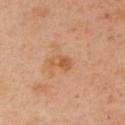Captured during whole-body skin photography for melanoma surveillance; the lesion was not biopsied.
The total-body-photography lesion software estimated an outline eccentricity of about 0.75 (0 = round, 1 = elongated). The analysis additionally found an average lesion color of about L≈58 a*≈24 b*≈38 (CIELAB), roughly 8 lightness units darker than nearby skin, and a lesion-to-skin contrast of about 6 (normalized; higher = more distinct). The analysis additionally found a border-irregularity rating of about 3/10, a within-lesion color-variation index near 2.5/10, and peripheral color asymmetry of about 1.
The lesion's longest dimension is about 2.5 mm.
A female subject, aged around 40.
Located on the arm.
Imaged with cross-polarized lighting.
Cropped from a whole-body photographic skin survey; the tile spans about 15 mm.